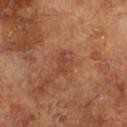Recorded during total-body skin imaging; not selected for excision or biopsy. Imaged with cross-polarized lighting. The recorded lesion diameter is about 2.5 mm. Located on the right lower leg. A male subject, aged 63 to 67. This image is a 15 mm lesion crop taken from a total-body photograph.